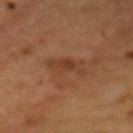Q: Was this lesion biopsied?
A: total-body-photography surveillance lesion; no biopsy
Q: Patient demographics?
A: female, aged 48 to 52
Q: Where on the body is the lesion?
A: the mid back
Q: What kind of image is this?
A: ~15 mm crop, total-body skin-cancer survey